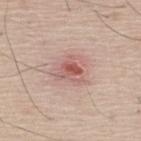No biopsy was performed on this lesion — it was imaged during a full skin examination and was not determined to be concerning.
The lesion's longest dimension is about 3 mm.
A male patient aged around 75.
On the upper back.
A 15 mm crop from a total-body photograph taken for skin-cancer surveillance.
Captured under white-light illumination.